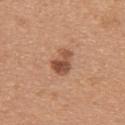The lesion is on the upper back. A female patient in their mid-30s. This image is a 15 mm lesion crop taken from a total-body photograph.